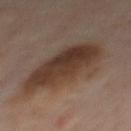Image and clinical context:
On the mid back. A 15 mm close-up extracted from a 3D total-body photography capture. The patient is a male aged approximately 65. Longest diameter approximately 8 mm. Automated tile analysis of the lesion measured a lesion area of about 33 mm². The analysis additionally found a lesion color around L≈35 a*≈15 b*≈23 in CIELAB, roughly 10 lightness units darker than nearby skin, and a lesion-to-skin contrast of about 9.5 (normalized; higher = more distinct).